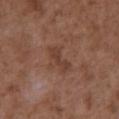notes=no biopsy performed (imaged during a skin exam)
size=about 3.5 mm
subject=male, aged around 75
anatomic site=the abdomen
imaging modality=total-body-photography crop, ~15 mm field of view